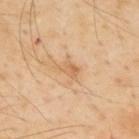workup = no biopsy performed (imaged during a skin exam)
image = 15 mm crop, total-body photography
lighting = cross-polarized
subject = male, about 55 years old
anatomic site = the upper back